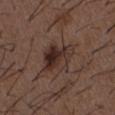Context:
Automated image analysis of the tile measured a nevus-likeness score of about 50/100 and a lesion-detection confidence of about 100/100. Captured under white-light illumination. Cropped from a whole-body photographic skin survey; the tile spans about 15 mm. A male patient, approximately 50 years of age. The recorded lesion diameter is about 5 mm. Located on the chest.